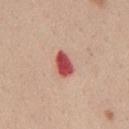Case summary:
• biopsy status — total-body-photography surveillance lesion; no biopsy
• patient — female, approximately 40 years of age
• acquisition — ~15 mm tile from a whole-body skin photo
• lesion size — about 3 mm
• tile lighting — white-light
• body site — the mid back